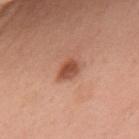Case summary:
* follow-up — imaged on a skin check; not biopsied
* TBP lesion metrics — an average lesion color of about L≈51 a*≈26 b*≈32 (CIELAB) and a lesion-to-skin contrast of about 8.5 (normalized; higher = more distinct); a border-irregularity index near 2/10, a within-lesion color-variation index near 3.5/10, and peripheral color asymmetry of about 1; an automated nevus-likeness rating near 90 out of 100 and a detector confidence of about 100 out of 100 that the crop contains a lesion
* image — 15 mm crop, total-body photography
* illumination — white-light
* anatomic site — the upper back
* subject — female, in their 50s
* lesion diameter — about 3 mm